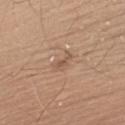The lesion was tiled from a total-body skin photograph and was not biopsied. A male subject aged 43 to 47. This image is a 15 mm lesion crop taken from a total-body photograph. Automated image analysis of the tile measured a footprint of about 3.5 mm², an eccentricity of roughly 0.9, and a symmetry-axis asymmetry near 0.35. The analysis additionally found a border-irregularity index near 4/10 and a peripheral color-asymmetry measure near 0.5. Located on the upper back. Longest diameter approximately 3 mm.